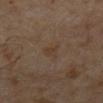| key | value |
|---|---|
| biopsy status | no biopsy performed (imaged during a skin exam) |
| patient | male, aged 63 to 67 |
| imaging modality | total-body-photography crop, ~15 mm field of view |
| diameter | ≈3 mm |